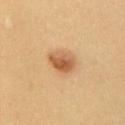Imaged during a routine full-body skin examination; the lesion was not biopsied and no histopathology is available.
The recorded lesion diameter is about 3 mm.
On the front of the torso.
A male patient, about 55 years old.
A roughly 15 mm field-of-view crop from a total-body skin photograph.